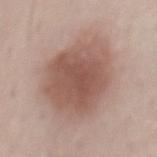{
  "biopsy_status": "not biopsied; imaged during a skin examination",
  "site": "mid back",
  "image": {
    "source": "total-body photography crop",
    "field_of_view_mm": 15
  },
  "lighting": "white-light",
  "patient": {
    "sex": "male",
    "age_approx": 75
  }
}